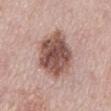Q: Is there a histopathology result?
A: catalogued during a skin exam; not biopsied
Q: Lesion location?
A: the mid back
Q: What are the patient's age and sex?
A: male, aged 73–77
Q: What is the imaging modality?
A: ~15 mm tile from a whole-body skin photo